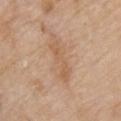The lesion was tiled from a total-body skin photograph and was not biopsied.
The patient is a male in their 80s.
From the upper back.
Captured under white-light illumination.
A lesion tile, about 15 mm wide, cut from a 3D total-body photograph.
The recorded lesion diameter is about 4.5 mm.